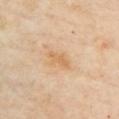Context:
On the chest. A female subject, aged 58 to 62. Cropped from a total-body skin-imaging series; the visible field is about 15 mm. Imaged with cross-polarized lighting.